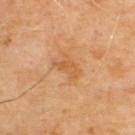  biopsy_status: not biopsied; imaged during a skin examination
  lighting: cross-polarized
  site: upper back
  patient:
    sex: male
    age_approx: 70
  image:
    source: total-body photography crop
    field_of_view_mm: 15
  lesion_size:
    long_diameter_mm_approx: 3.5